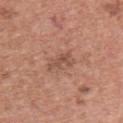Clinical impression: Recorded during total-body skin imaging; not selected for excision or biopsy. Background: Automated image analysis of the tile measured a border-irregularity index near 5.5/10, a color-variation rating of about 0.5/10, and radial color variation of about 0. Located on the upper back. The patient is a female roughly 60 years of age. A 15 mm close-up extracted from a 3D total-body photography capture. Approximately 3.5 mm at its widest.